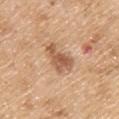| key | value |
|---|---|
| notes | catalogued during a skin exam; not biopsied |
| anatomic site | the upper back |
| image | 15 mm crop, total-body photography |
| subject | male, aged 68 to 72 |